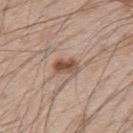<tbp_lesion>
<patient>
  <sex>male</sex>
  <age_approx>50</age_approx>
</patient>
<site>upper back</site>
<lighting>white-light</lighting>
<image>
  <source>total-body photography crop</source>
  <field_of_view_mm>15</field_of_view_mm>
</image>
<lesion_size>
  <long_diameter_mm_approx>3.0</long_diameter_mm_approx>
</lesion_size>
</tbp_lesion>